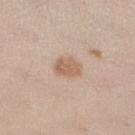Recorded during total-body skin imaging; not selected for excision or biopsy.
The lesion is located on the right lower leg.
A female subject aged 38–42.
This image is a 15 mm lesion crop taken from a total-body photograph.
Measured at roughly 3 mm in maximum diameter.
Imaged with white-light lighting.